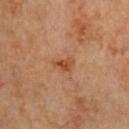A lesion tile, about 15 mm wide, cut from a 3D total-body photograph.
Imaged with cross-polarized lighting.
The total-body-photography lesion software estimated a shape eccentricity near 0.75 and a shape-asymmetry score of about 0.3 (0 = symmetric). The analysis additionally found a within-lesion color-variation index near 3/10 and peripheral color asymmetry of about 1. It also reported a nevus-likeness score of about 0/100 and a detector confidence of about 100 out of 100 that the crop contains a lesion.
The patient is female.
The lesion is on the chest.